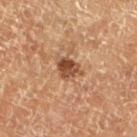Context:
A male patient, approximately 75 years of age. From the left lower leg. A lesion tile, about 15 mm wide, cut from a 3D total-body photograph.